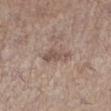Case summary:
* notes: imaged on a skin check; not biopsied
* image source: ~15 mm tile from a whole-body skin photo
* diameter: ~3.5 mm (longest diameter)
* subject: female, roughly 45 years of age
* tile lighting: white-light
* body site: the left lower leg